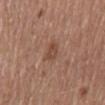{"biopsy_status": "not biopsied; imaged during a skin examination", "automated_metrics": {"cielab_L": 46, "cielab_a": 21, "cielab_b": 28, "vs_skin_darker_L": 8.0, "vs_skin_contrast_norm": 6.0, "border_irregularity_0_10": 3.0, "color_variation_0_10": 3.5}, "image": {"source": "total-body photography crop", "field_of_view_mm": 15}, "lighting": "white-light", "patient": {"sex": "male", "age_approx": 65}, "site": "back"}